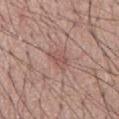Assessment: Captured during whole-body skin photography for melanoma surveillance; the lesion was not biopsied. Image and clinical context: From the chest. A close-up tile cropped from a whole-body skin photograph, about 15 mm across. The total-body-photography lesion software estimated a mean CIELAB color near L≈53 a*≈22 b*≈23 and a lesion–skin lightness drop of about 7. And it measured a border-irregularity rating of about 3.5/10, internal color variation of about 2.5 on a 0–10 scale, and peripheral color asymmetry of about 1. Approximately 3 mm at its widest. Captured under white-light illumination. The patient is a male aged around 65.